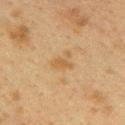workup = imaged on a skin check; not biopsied | site = the upper back | image = 15 mm crop, total-body photography | subject = female, aged 38–42.